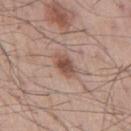{"biopsy_status": "not biopsied; imaged during a skin examination", "lesion_size": {"long_diameter_mm_approx": 3.5}, "automated_metrics": {"area_mm2_approx": 5.5, "eccentricity": 0.8, "shape_asymmetry": 0.15, "cielab_L": 51, "cielab_a": 20, "cielab_b": 26, "vs_skin_darker_L": 12.0, "vs_skin_contrast_norm": 8.5}, "image": {"source": "total-body photography crop", "field_of_view_mm": 15}, "site": "mid back", "patient": {"sex": "male", "age_approx": 55}, "lighting": "white-light"}